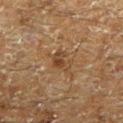Impression: Imaged during a routine full-body skin examination; the lesion was not biopsied and no histopathology is available. Image and clinical context: The lesion is located on the left lower leg. A roughly 15 mm field-of-view crop from a total-body skin photograph. The subject is a male approximately 60 years of age. This is a cross-polarized tile. The lesion's longest dimension is about 3 mm. The lesion-visualizer software estimated a lesion area of about 5 mm², an eccentricity of roughly 0.75, and two-axis asymmetry of about 0.3. It also reported a classifier nevus-likeness of about 0/100 and a lesion-detection confidence of about 95/100.